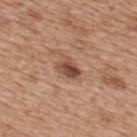<lesion>
  <patient>
    <sex>male</sex>
    <age_approx>65</age_approx>
  </patient>
  <image>
    <source>total-body photography crop</source>
    <field_of_view_mm>15</field_of_view_mm>
  </image>
  <lighting>white-light</lighting>
  <site>upper back</site>
</lesion>